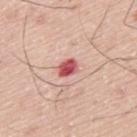Captured during whole-body skin photography for melanoma surveillance; the lesion was not biopsied. A close-up tile cropped from a whole-body skin photograph, about 15 mm across. The lesion is on the upper back. Approximately 2.5 mm at its widest. Imaged with white-light lighting. A male patient in their mid- to late 70s.